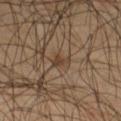Notes:
• notes · imaged on a skin check; not biopsied
• acquisition · ~15 mm crop, total-body skin-cancer survey
• lighting · cross-polarized
• size · ≈2.5 mm
• body site · the right thigh
• subject · male, in their mid-50s